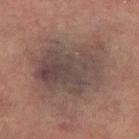Case summary:
– notes: no biopsy performed (imaged during a skin exam)
– subject: female, aged 58–62
– image source: 15 mm crop, total-body photography
– image-analysis metrics: an outline eccentricity of about 0.7 (0 = round, 1 = elongated); a lesion color around L≈38 a*≈13 b*≈17 in CIELAB, roughly 8 lightness units darker than nearby skin, and a normalized border contrast of about 8; border irregularity of about 4 on a 0–10 scale, a color-variation rating of about 5/10, and radial color variation of about 1.5; an automated nevus-likeness rating near 0 out of 100 and a lesion-detection confidence of about 95/100
– diameter: about 8 mm
– body site: the left thigh
– lighting: cross-polarized illumination